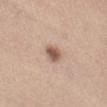{"biopsy_status": "not biopsied; imaged during a skin examination", "patient": {"sex": "female", "age_approx": 45}, "site": "left forearm", "lighting": "white-light", "automated_metrics": {"nevus_likeness_0_100": 90, "lesion_detection_confidence_0_100": 100}, "lesion_size": {"long_diameter_mm_approx": 2.5}, "image": {"source": "total-body photography crop", "field_of_view_mm": 15}}